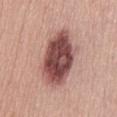<tbp_lesion>
<biopsy_status>not biopsied; imaged during a skin examination</biopsy_status>
<site>abdomen</site>
<image>
  <source>total-body photography crop</source>
  <field_of_view_mm>15</field_of_view_mm>
</image>
<patient>
  <sex>male</sex>
  <age_approx>65</age_approx>
</patient>
</tbp_lesion>